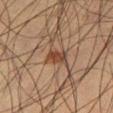{
  "biopsy_status": "not biopsied; imaged during a skin examination",
  "site": "leg",
  "lighting": "cross-polarized",
  "patient": {
    "sex": "male",
    "age_approx": 50
  },
  "image": {
    "source": "total-body photography crop",
    "field_of_view_mm": 15
  }
}